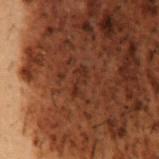Assessment:
This lesion was catalogued during total-body skin photography and was not selected for biopsy.
Background:
The tile uses cross-polarized illumination. The patient is a male roughly 50 years of age. The recorded lesion diameter is about 3 mm. Cropped from a whole-body photographic skin survey; the tile spans about 15 mm. The lesion is located on the abdomen.